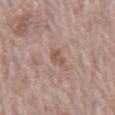<tbp_lesion>
<biopsy_status>not biopsied; imaged during a skin examination</biopsy_status>
<patient>
  <sex>male</sex>
  <age_approx>70</age_approx>
</patient>
<lesion_size>
  <long_diameter_mm_approx>3.0</long_diameter_mm_approx>
</lesion_size>
<automated_metrics>
  <area_mm2_approx>3.0</area_mm2_approx>
  <eccentricity>0.9</eccentricity>
  <shape_asymmetry>0.45</shape_asymmetry>
  <cielab_L>54</cielab_L>
  <cielab_a>19</cielab_a>
  <cielab_b>26</cielab_b>
  <vs_skin_darker_L>8.0</vs_skin_darker_L>
  <vs_skin_contrast_norm>6.0</vs_skin_contrast_norm>
</automated_metrics>
<lighting>white-light</lighting>
<site>front of the torso</site>
<image>
  <source>total-body photography crop</source>
  <field_of_view_mm>15</field_of_view_mm>
</image>
</tbp_lesion>